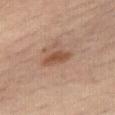The lesion was photographed on a routine skin check and not biopsied; there is no pathology result. A male patient, aged 58 to 62. The lesion is located on the leg. A close-up tile cropped from a whole-body skin photograph, about 15 mm across. Approximately 3.5 mm at its widest.